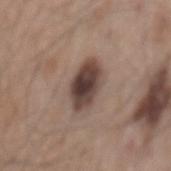Impression:
The lesion was photographed on a routine skin check and not biopsied; there is no pathology result.
Clinical summary:
A male patient, in their mid-50s. On the mid back. A lesion tile, about 15 mm wide, cut from a 3D total-body photograph. This is a white-light tile. About 5 mm across. The total-body-photography lesion software estimated border irregularity of about 2 on a 0–10 scale, internal color variation of about 6 on a 0–10 scale, and peripheral color asymmetry of about 1.5.